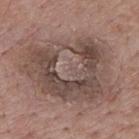notes: catalogued during a skin exam; not biopsied | diameter: about 12 mm | site: the upper back | image source: ~15 mm tile from a whole-body skin photo | patient: male, about 70 years old | illumination: white-light illumination.